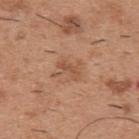Captured during whole-body skin photography for melanoma surveillance; the lesion was not biopsied. A 15 mm crop from a total-body photograph taken for skin-cancer surveillance. A male subject in their 40s. On the upper back.